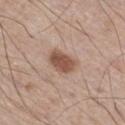| field | value |
|---|---|
| notes | imaged on a skin check; not biopsied |
| diameter | about 3 mm |
| patient | male, aged 58–62 |
| anatomic site | the leg |
| tile lighting | white-light illumination |
| image source | ~15 mm tile from a whole-body skin photo |